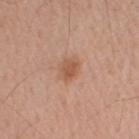Findings:
- notes: no biopsy performed (imaged during a skin exam)
- anatomic site: the right upper arm
- diameter: ≈2.5 mm
- acquisition: total-body-photography crop, ~15 mm field of view
- automated lesion analysis: a lesion color around L≈55 a*≈23 b*≈32 in CIELAB, about 9 CIELAB-L* units darker than the surrounding skin, and a lesion-to-skin contrast of about 7 (normalized; higher = more distinct); border irregularity of about 2 on a 0–10 scale and peripheral color asymmetry of about 0.5; a classifier nevus-likeness of about 75/100 and a detector confidence of about 100 out of 100 that the crop contains a lesion
- subject: male, aged approximately 60
- tile lighting: white-light